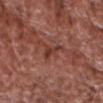Q: Was a biopsy performed?
A: imaged on a skin check; not biopsied
Q: What is the lesion's diameter?
A: ~2.5 mm (longest diameter)
Q: How was this image acquired?
A: ~15 mm tile from a whole-body skin photo
Q: Lesion location?
A: the head or neck
Q: Who is the patient?
A: male, aged approximately 75
Q: What lighting was used for the tile?
A: white-light illumination